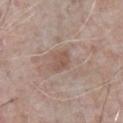Case summary:
* workup: no biopsy performed (imaged during a skin exam)
* acquisition: ~15 mm tile from a whole-body skin photo
* anatomic site: the front of the torso
* diameter: ≈2.5 mm
* subject: male, aged around 65